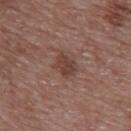Findings:
– notes · imaged on a skin check; not biopsied
– tile lighting · white-light
– site · the upper back
– imaging modality · ~15 mm crop, total-body skin-cancer survey
– lesion size · ≈3 mm
– TBP lesion metrics · an area of roughly 5.5 mm² and an eccentricity of roughly 0.6
– patient · female, in their mid- to late 60s